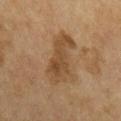biopsy status=imaged on a skin check; not biopsied
image source=total-body-photography crop, ~15 mm field of view
subject=female, aged around 60
body site=the chest
size=≈6.5 mm
automated lesion analysis=an average lesion color of about L≈41 a*≈15 b*≈31 (CIELAB), a lesion–skin lightness drop of about 8, and a normalized lesion–skin contrast near 7; an automated nevus-likeness rating near 5 out of 100 and lesion-presence confidence of about 100/100